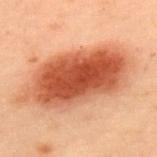Context: This image is a 15 mm lesion crop taken from a total-body photograph. The total-body-photography lesion software estimated a border-irregularity index near 2.5/10, a within-lesion color-variation index near 7.5/10, and a peripheral color-asymmetry measure near 2. It also reported lesion-presence confidence of about 100/100. Imaged with cross-polarized lighting. Longest diameter approximately 12 mm. The lesion is on the upper back. A male subject in their mid-50s.